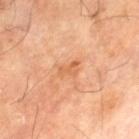Q: Was a biopsy performed?
A: imaged on a skin check; not biopsied
Q: Lesion location?
A: the left thigh
Q: What is the lesion's diameter?
A: about 2.5 mm
Q: Patient demographics?
A: male, approximately 70 years of age
Q: What did automated image analysis measure?
A: an average lesion color of about L≈62 a*≈26 b*≈41 (CIELAB); an automated nevus-likeness rating near 0 out of 100 and lesion-presence confidence of about 100/100
Q: What kind of image is this?
A: ~15 mm tile from a whole-body skin photo